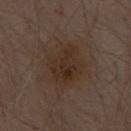The lesion was tiled from a total-body skin photograph and was not biopsied.
Located on the abdomen.
Longest diameter approximately 4.5 mm.
The lesion-visualizer software estimated a border-irregularity index near 2/10, internal color variation of about 4 on a 0–10 scale, and peripheral color asymmetry of about 1.5. The software also gave a nevus-likeness score of about 10/100.
A 15 mm crop from a total-body photograph taken for skin-cancer surveillance.
A male subject aged 68–72.